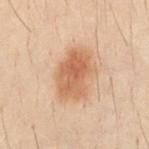{"biopsy_status": "not biopsied; imaged during a skin examination", "site": "chest", "patient": {"sex": "male", "age_approx": 30}, "image": {"source": "total-body photography crop", "field_of_view_mm": 15}}